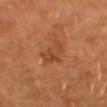Clinical impression:
This lesion was catalogued during total-body skin photography and was not selected for biopsy.
Clinical summary:
An algorithmic analysis of the crop reported an eccentricity of roughly 0.75. It also reported a mean CIELAB color near L≈43 a*≈25 b*≈34 and a lesion-to-skin contrast of about 5.5 (normalized; higher = more distinct). It also reported a border-irregularity rating of about 4.5/10 and a within-lesion color-variation index near 4/10. The analysis additionally found a classifier nevus-likeness of about 5/100 and a detector confidence of about 100 out of 100 that the crop contains a lesion. A region of skin cropped from a whole-body photographic capture, roughly 15 mm wide. On the head or neck. A male patient, aged 58 to 62. Captured under cross-polarized illumination. The recorded lesion diameter is about 3.5 mm.